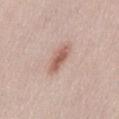Recorded during total-body skin imaging; not selected for excision or biopsy. An algorithmic analysis of the crop reported a lesion area of about 5 mm² and an eccentricity of roughly 0.9. The analysis additionally found a mean CIELAB color near L≈58 a*≈21 b*≈26, roughly 12 lightness units darker than nearby skin, and a normalized border contrast of about 8. The software also gave a border-irregularity index near 2.5/10, internal color variation of about 2.5 on a 0–10 scale, and radial color variation of about 0.5. A 15 mm close-up extracted from a 3D total-body photography capture. The subject is a female aged 28–32. The lesion's longest dimension is about 4 mm. From the front of the torso.